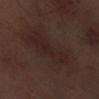Q: Was a biopsy performed?
A: total-body-photography surveillance lesion; no biopsy
Q: What is the anatomic site?
A: the leg
Q: Patient demographics?
A: male, roughly 70 years of age
Q: How was this image acquired?
A: ~15 mm tile from a whole-body skin photo